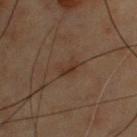biopsy_status: not biopsied; imaged during a skin examination
patient:
  sex: male
  age_approx: 55
image:
  source: total-body photography crop
  field_of_view_mm: 15
lighting: cross-polarized
lesion_size:
  long_diameter_mm_approx: 2.5
site: chest